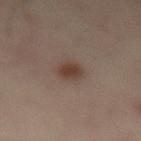Assessment:
No biopsy was performed on this lesion — it was imaged during a full skin examination and was not determined to be concerning.